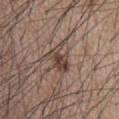Notes:
* notes: imaged on a skin check; not biopsied
* location: the chest
* diameter: ≈4 mm
* illumination: white-light
* subject: male, about 55 years old
* automated lesion analysis: a mean CIELAB color near L≈43 a*≈16 b*≈22 and a normalized lesion–skin contrast near 9; a border-irregularity index near 4/10 and a color-variation rating of about 4/10; an automated nevus-likeness rating near 10 out of 100 and a lesion-detection confidence of about 95/100
* image: 15 mm crop, total-body photography